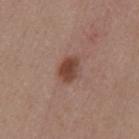Assessment: No biopsy was performed on this lesion — it was imaged during a full skin examination and was not determined to be concerning. Clinical summary: A 15 mm crop from a total-body photograph taken for skin-cancer surveillance. An algorithmic analysis of the crop reported a footprint of about 6 mm², an eccentricity of roughly 0.65, and a symmetry-axis asymmetry near 0.2. And it measured a lesion color around L≈44 a*≈21 b*≈26 in CIELAB and a lesion-to-skin contrast of about 9.5 (normalized; higher = more distinct). The analysis additionally found a border-irregularity index near 2/10, internal color variation of about 3.5 on a 0–10 scale, and peripheral color asymmetry of about 1. It also reported a classifier nevus-likeness of about 95/100 and a lesion-detection confidence of about 100/100. A female patient, aged 43–47. Located on the mid back. This is a white-light tile.